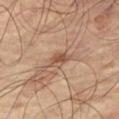No biopsy was performed on this lesion — it was imaged during a full skin examination and was not determined to be concerning. About 3 mm across. This is a cross-polarized tile. Automated tile analysis of the lesion measured a lesion area of about 4.5 mm² and a symmetry-axis asymmetry near 0.35. A 15 mm close-up extracted from a 3D total-body photography capture. A male patient roughly 65 years of age. On the right thigh.